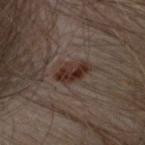No biopsy was performed on this lesion — it was imaged during a full skin examination and was not determined to be concerning. On the head or neck. The patient is a female roughly 30 years of age. Automated tile analysis of the lesion measured a lesion color around L≈23 a*≈13 b*≈17 in CIELAB. And it measured border irregularity of about 2 on a 0–10 scale, a color-variation rating of about 4.5/10, and radial color variation of about 1.5. And it measured a nevus-likeness score of about 85/100 and a lesion-detection confidence of about 100/100. About 3.5 mm across. This image is a 15 mm lesion crop taken from a total-body photograph. This is a cross-polarized tile.